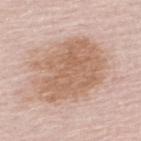Captured during whole-body skin photography for melanoma surveillance; the lesion was not biopsied. Captured under white-light illumination. A male patient, aged around 65. A lesion tile, about 15 mm wide, cut from a 3D total-body photograph. The lesion is on the upper back. The recorded lesion diameter is about 8.5 mm.